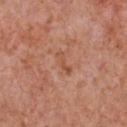{
  "biopsy_status": "not biopsied; imaged during a skin examination"
}